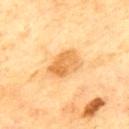The lesion was photographed on a routine skin check and not biopsied; there is no pathology result. The tile uses cross-polarized illumination. A region of skin cropped from a whole-body photographic capture, roughly 15 mm wide. From the upper back. A male subject aged 68–72.